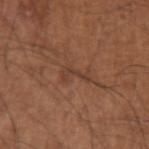The lesion was tiled from a total-body skin photograph and was not biopsied.
A male subject, about 65 years old.
From the left upper arm.
This is a white-light tile.
A 15 mm close-up extracted from a 3D total-body photography capture.
The lesion's longest dimension is about 3.5 mm.
The lesion-visualizer software estimated a mean CIELAB color near L≈40 a*≈20 b*≈28, a lesion–skin lightness drop of about 6, and a lesion-to-skin contrast of about 5.5 (normalized; higher = more distinct). The software also gave a classifier nevus-likeness of about 0/100 and a detector confidence of about 80 out of 100 that the crop contains a lesion.